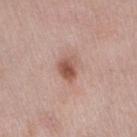Recorded during total-body skin imaging; not selected for excision or biopsy. The recorded lesion diameter is about 3 mm. The total-body-photography lesion software estimated a border-irregularity rating of about 2.5/10 and internal color variation of about 5.5 on a 0–10 scale. Located on the right thigh. Captured under white-light illumination. The patient is a female aged approximately 40. This image is a 15 mm lesion crop taken from a total-body photograph.